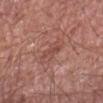  biopsy_status: not biopsied; imaged during a skin examination
  lesion_size:
    long_diameter_mm_approx: 3.5
  patient:
    sex: male
    age_approx: 65
  site: arm
  lighting: white-light
  image:
    source: total-body photography crop
    field_of_view_mm: 15
  automated_metrics:
    cielab_L: 49
    cielab_a: 24
    cielab_b: 25
    vs_skin_contrast_norm: 5.0
    border_irregularity_0_10: 2.5
    color_variation_0_10: 3.5
    peripheral_color_asymmetry: 1.0